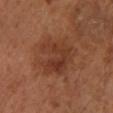The lesion was photographed on a routine skin check and not biopsied; there is no pathology result. A roughly 15 mm field-of-view crop from a total-body skin photograph. A female subject approximately 65 years of age. The lesion is on the left lower leg.